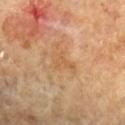{
  "biopsy_status": "not biopsied; imaged during a skin examination",
  "image": {
    "source": "total-body photography crop",
    "field_of_view_mm": 15
  },
  "site": "left forearm",
  "patient": {
    "sex": "female",
    "age_approx": 65
  },
  "automated_metrics": {
    "area_mm2_approx": 5.0,
    "shape_asymmetry": 0.45,
    "nevus_likeness_0_100": 0,
    "lesion_detection_confidence_0_100": 100
  }
}